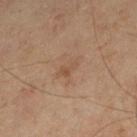Recorded during total-body skin imaging; not selected for excision or biopsy.
A male subject approximately 70 years of age.
Cropped from a total-body skin-imaging series; the visible field is about 15 mm.
This is a cross-polarized tile.
The lesion is on the left lower leg.